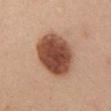<tbp_lesion>
  <biopsy_status>not biopsied; imaged during a skin examination</biopsy_status>
  <site>chest</site>
  <lesion_size>
    <long_diameter_mm_approx>6.0</long_diameter_mm_approx>
  </lesion_size>
  <patient>
    <sex>female</sex>
    <age_approx>25</age_approx>
  </patient>
  <lighting>white-light</lighting>
  <image>
    <source>total-body photography crop</source>
    <field_of_view_mm>15</field_of_view_mm>
  </image>
  <automated_metrics>
    <area_mm2_approx>24.0</area_mm2_approx>
    <eccentricity>0.6</eccentricity>
    <border_irregularity_0_10>1.0</border_irregularity_0_10>
    <color_variation_0_10>5.5</color_variation_0_10>
    <peripheral_color_asymmetry>1.5</peripheral_color_asymmetry>
    <nevus_likeness_0_100>100</nevus_likeness_0_100>
    <lesion_detection_confidence_0_100>100</lesion_detection_confidence_0_100>
  </automated_metrics>
</tbp_lesion>